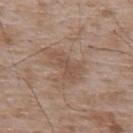The lesion was tiled from a total-body skin photograph and was not biopsied. A male patient, in their 50s. From the upper back. A roughly 15 mm field-of-view crop from a total-body skin photograph.